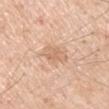Part of a total-body skin-imaging series; this lesion was reviewed on a skin check and was not flagged for biopsy.
The recorded lesion diameter is about 3.5 mm.
A male patient, approximately 60 years of age.
A close-up tile cropped from a whole-body skin photograph, about 15 mm across.
Automated tile analysis of the lesion measured border irregularity of about 3.5 on a 0–10 scale, internal color variation of about 2.5 on a 0–10 scale, and a peripheral color-asymmetry measure near 1.
The lesion is on the left upper arm.